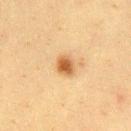| key | value |
|---|---|
| image source | 15 mm crop, total-body photography |
| illumination | cross-polarized illumination |
| patient | female, aged 38–42 |
| site | the chest |
| diameter | about 2.5 mm |